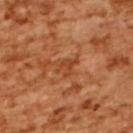workup = total-body-photography surveillance lesion; no biopsy | tile lighting = cross-polarized | anatomic site = the upper back | image = 15 mm crop, total-body photography | lesion size = ~3 mm (longest diameter) | subject = female, aged around 55 | automated lesion analysis = a lesion color around L≈47 a*≈29 b*≈40 in CIELAB, roughly 7 lightness units darker than nearby skin, and a normalized border contrast of about 6.